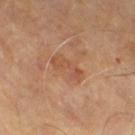Part of a total-body skin-imaging series; this lesion was reviewed on a skin check and was not flagged for biopsy.
A 15 mm crop from a total-body photograph taken for skin-cancer surveillance.
A patient about 65 years old.
On the right thigh.
Captured under cross-polarized illumination.